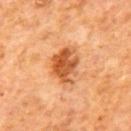Q: Was this lesion biopsied?
A: total-body-photography surveillance lesion; no biopsy
Q: Lesion location?
A: the back
Q: What is the lesion's diameter?
A: ≈4.5 mm
Q: What kind of image is this?
A: ~15 mm tile from a whole-body skin photo
Q: How was the tile lit?
A: cross-polarized illumination
Q: Who is the patient?
A: male, in their mid-60s